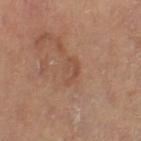diameter: about 3 mm | image-analysis metrics: a lesion color around L≈49 a*≈20 b*≈29 in CIELAB and a normalized lesion–skin contrast near 4.5 | anatomic site: the left thigh | patient: female, about 65 years old | illumination: cross-polarized | imaging modality: total-body-photography crop, ~15 mm field of view.